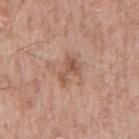Recorded during total-body skin imaging; not selected for excision or biopsy. The tile uses white-light illumination. The lesion is located on the mid back. A male patient, about 55 years old. About 3.5 mm across. A lesion tile, about 15 mm wide, cut from a 3D total-body photograph. Automated image analysis of the tile measured an area of roughly 4.5 mm². And it measured a mean CIELAB color near L≈54 a*≈21 b*≈29, about 9 CIELAB-L* units darker than the surrounding skin, and a normalized border contrast of about 6.5. And it measured a classifier nevus-likeness of about 0/100 and lesion-presence confidence of about 100/100.